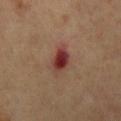Recorded during total-body skin imaging; not selected for excision or biopsy. On the chest. The patient is a male aged 68 to 72. Measured at roughly 3.5 mm in maximum diameter. A lesion tile, about 15 mm wide, cut from a 3D total-body photograph. This is a cross-polarized tile.